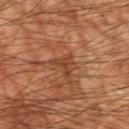Part of a total-body skin-imaging series; this lesion was reviewed on a skin check and was not flagged for biopsy.
The recorded lesion diameter is about 3 mm.
The patient is a male about 60 years old.
Located on the right upper arm.
The tile uses cross-polarized illumination.
Cropped from a whole-body photographic skin survey; the tile spans about 15 mm.